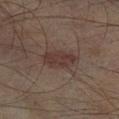| field | value |
|---|---|
| workup | catalogued during a skin exam; not biopsied |
| tile lighting | cross-polarized |
| lesion diameter | about 4 mm |
| anatomic site | the left lower leg |
| imaging modality | total-body-photography crop, ~15 mm field of view |
| patient | male, aged 73–77 |